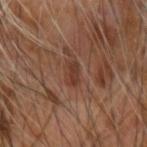Q: Was this lesion biopsied?
A: catalogued during a skin exam; not biopsied
Q: How large is the lesion?
A: about 2.5 mm
Q: What did automated image analysis measure?
A: an area of roughly 3 mm², a shape eccentricity near 0.85, and a symmetry-axis asymmetry near 0.2; about 8 CIELAB-L* units darker than the surrounding skin and a normalized lesion–skin contrast near 7
Q: How was this image acquired?
A: ~15 mm crop, total-body skin-cancer survey
Q: Lesion location?
A: the right forearm
Q: How was the tile lit?
A: cross-polarized
Q: Who is the patient?
A: male, aged approximately 65